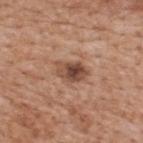Captured during whole-body skin photography for melanoma surveillance; the lesion was not biopsied. The lesion is on the upper back. A male subject in their mid-60s. Approximately 3.5 mm at its widest. A 15 mm crop from a total-body photograph taken for skin-cancer surveillance. This is a white-light tile.